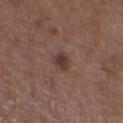Context: A close-up tile cropped from a whole-body skin photograph, about 15 mm across. The lesion's longest dimension is about 3 mm. The patient is a female aged 33 to 37. The tile uses white-light illumination. On the upper back.